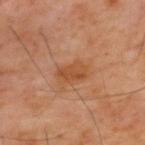Assessment:
Captured during whole-body skin photography for melanoma surveillance; the lesion was not biopsied.
Background:
Captured under cross-polarized illumination. Measured at roughly 3.5 mm in maximum diameter. From the mid back. A 15 mm close-up tile from a total-body photography series done for melanoma screening. A male patient, approximately 60 years of age. An algorithmic analysis of the crop reported a lesion color around L≈46 a*≈23 b*≈36 in CIELAB and a normalized border contrast of about 7. The analysis additionally found a border-irregularity index near 2.5/10, internal color variation of about 2.5 on a 0–10 scale, and radial color variation of about 1. The analysis additionally found a classifier nevus-likeness of about 0/100.